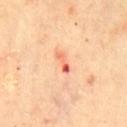The total-body-photography lesion software estimated border irregularity of about 5 on a 0–10 scale, a color-variation rating of about 0/10, and a peripheral color-asymmetry measure near 0.
Captured under cross-polarized illumination.
From the front of the torso.
The lesion's longest dimension is about 2.5 mm.
A male subject, about 65 years old.
This image is a 15 mm lesion crop taken from a total-body photograph.